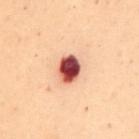Part of a total-body skin-imaging series; this lesion was reviewed on a skin check and was not flagged for biopsy. Cropped from a whole-body photographic skin survey; the tile spans about 15 mm. Approximately 3.5 mm at its widest. From the back. Captured under cross-polarized illumination. A female subject, aged 48 to 52. The lesion-visualizer software estimated a footprint of about 7.5 mm² and a shape-asymmetry score of about 0.15 (0 = symmetric).